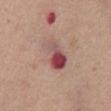No biopsy was performed on this lesion — it was imaged during a full skin examination and was not determined to be concerning. A male patient, about 55 years old. Automated image analysis of the tile measured a border-irregularity index near 3.5/10. Cropped from a total-body skin-imaging series; the visible field is about 15 mm. The tile uses white-light illumination. Located on the abdomen.